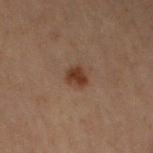| feature | finding |
|---|---|
| workup | imaged on a skin check; not biopsied |
| image source | ~15 mm tile from a whole-body skin photo |
| automated metrics | a footprint of about 4.5 mm², a shape eccentricity near 0.55, and a symmetry-axis asymmetry near 0.25; a lesion color around L≈27 a*≈15 b*≈22 in CIELAB and roughly 8 lightness units darker than nearby skin; peripheral color asymmetry of about 0.5; a nevus-likeness score of about 95/100 |
| subject | male, aged 68 to 72 |
| illumination | cross-polarized |
| body site | the arm |